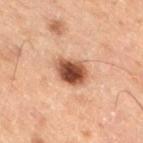biopsy status: no biopsy performed (imaged during a skin exam)
size: ~3.5 mm (longest diameter)
site: the leg
lighting: cross-polarized illumination
subject: male, roughly 70 years of age
acquisition: ~15 mm crop, total-body skin-cancer survey